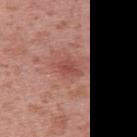workup: total-body-photography surveillance lesion; no biopsy
subject: female, aged 38–42
site: the upper back
lesion diameter: ~2.5 mm (longest diameter)
tile lighting: white-light
image: 15 mm crop, total-body photography